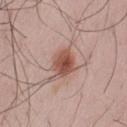Clinical impression:
This lesion was catalogued during total-body skin photography and was not selected for biopsy.
Image and clinical context:
A male patient aged 63 to 67. From the left thigh. A 15 mm crop from a total-body photograph taken for skin-cancer surveillance.